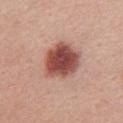This lesion was catalogued during total-body skin photography and was not selected for biopsy.